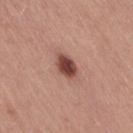Notes:
– workup: total-body-photography surveillance lesion; no biopsy
– site: the right thigh
– image source: ~15 mm crop, total-body skin-cancer survey
– automated metrics: an average lesion color of about L≈46 a*≈24 b*≈25 (CIELAB), a lesion–skin lightness drop of about 16, and a normalized lesion–skin contrast near 11; an automated nevus-likeness rating near 100 out of 100
– subject: female, in their mid-40s
– size: ~3.5 mm (longest diameter)